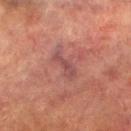The subject is a male aged around 75.
The lesion's longest dimension is about 4 mm.
A close-up tile cropped from a whole-body skin photograph, about 15 mm across.
From the leg.
Captured under cross-polarized illumination.